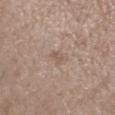The tile uses white-light illumination. A region of skin cropped from a whole-body photographic capture, roughly 15 mm wide. On the left forearm. A male patient, aged around 50. Longest diameter approximately 2.5 mm.